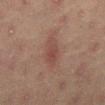Q: Is there a histopathology result?
A: no biopsy performed (imaged during a skin exam)
Q: What is the anatomic site?
A: the abdomen
Q: How was this image acquired?
A: ~15 mm tile from a whole-body skin photo
Q: Who is the patient?
A: male, roughly 45 years of age
Q: What did automated image analysis measure?
A: a lesion area of about 7 mm², a shape eccentricity near 0.9, and a shape-asymmetry score of about 0.2 (0 = symmetric); roughly 6 lightness units darker than nearby skin and a normalized lesion–skin contrast near 5.5; border irregularity of about 2.5 on a 0–10 scale, a color-variation rating of about 2.5/10, and a peripheral color-asymmetry measure near 1
Q: What lighting was used for the tile?
A: cross-polarized
Q: What is the lesion's diameter?
A: about 4.5 mm